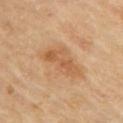Q: Was a biopsy performed?
A: imaged on a skin check; not biopsied
Q: What is the imaging modality?
A: total-body-photography crop, ~15 mm field of view
Q: Who is the patient?
A: female, aged approximately 70
Q: What lighting was used for the tile?
A: cross-polarized
Q: Where on the body is the lesion?
A: the upper back